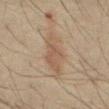follow-up: catalogued during a skin exam; not biopsied | illumination: cross-polarized | image source: 15 mm crop, total-body photography | site: the abdomen | patient: male, roughly 60 years of age | automated metrics: a mean CIELAB color near L≈45 a*≈13 b*≈25, roughly 6 lightness units darker than nearby skin, and a normalized lesion–skin contrast near 5.5; a border-irregularity rating of about 3.5/10 and radial color variation of about 1.